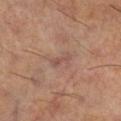{"biopsy_status": "not biopsied; imaged during a skin examination", "site": "leg", "patient": {"sex": "male", "age_approx": 60}, "image": {"source": "total-body photography crop", "field_of_view_mm": 15}}